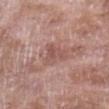Clinical impression: Imaged during a routine full-body skin examination; the lesion was not biopsied and no histopathology is available. Image and clinical context: A female patient, in their 70s. A region of skin cropped from a whole-body photographic capture, roughly 15 mm wide. On the left upper arm.